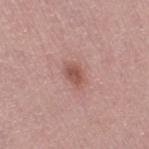Imaged during a routine full-body skin examination; the lesion was not biopsied and no histopathology is available.
The lesion is on the leg.
A female subject, aged around 60.
This is a white-light tile.
This image is a 15 mm lesion crop taken from a total-body photograph.
The recorded lesion diameter is about 3 mm.